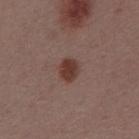Case summary:
- workup: imaged on a skin check; not biopsied